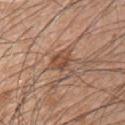The lesion was photographed on a routine skin check and not biopsied; there is no pathology result.
The recorded lesion diameter is about 3.5 mm.
The subject is a male in their 50s.
The lesion-visualizer software estimated a lesion area of about 6.5 mm², an outline eccentricity of about 0.3 (0 = round, 1 = elongated), and a symmetry-axis asymmetry near 0.55. And it measured border irregularity of about 7 on a 0–10 scale, a within-lesion color-variation index near 4.5/10, and radial color variation of about 1.5.
A region of skin cropped from a whole-body photographic capture, roughly 15 mm wide.
Imaged with white-light lighting.
From the chest.